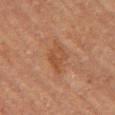Clinical impression: Part of a total-body skin-imaging series; this lesion was reviewed on a skin check and was not flagged for biopsy.